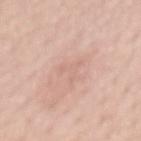Q: Is there a histopathology result?
A: imaged on a skin check; not biopsied
Q: Lesion size?
A: about 3.5 mm
Q: Patient demographics?
A: female, aged 53 to 57
Q: How was this image acquired?
A: ~15 mm crop, total-body skin-cancer survey
Q: Automated lesion metrics?
A: a shape eccentricity near 0.7 and a shape-asymmetry score of about 0.7 (0 = symmetric)
Q: Where on the body is the lesion?
A: the mid back